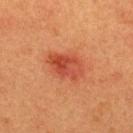Imaged during a routine full-body skin examination; the lesion was not biopsied and no histopathology is available. A male subject aged 38–42. The lesion is on the back. A 15 mm crop from a total-body photograph taken for skin-cancer surveillance. Captured under cross-polarized illumination. Longest diameter approximately 4.5 mm.